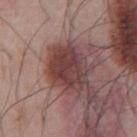The lesion was photographed on a routine skin check and not biopsied; there is no pathology result. From the chest. A region of skin cropped from a whole-body photographic capture, roughly 15 mm wide. A male patient, in their mid-50s.